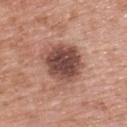- follow-up: catalogued during a skin exam; not biopsied
- image: total-body-photography crop, ~15 mm field of view
- lighting: white-light illumination
- anatomic site: the upper back
- subject: female, aged 58–62
- automated metrics: a footprint of about 18 mm² and an outline eccentricity of about 0.4 (0 = round, 1 = elongated); a border-irregularity rating of about 2.5/10 and a within-lesion color-variation index near 5/10